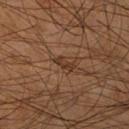| feature | finding |
|---|---|
| diameter | ≈2.5 mm |
| tile lighting | cross-polarized illumination |
| body site | the right lower leg |
| image-analysis metrics | an average lesion color of about L≈31 a*≈17 b*≈27 (CIELAB), about 8 CIELAB-L* units darker than the surrounding skin, and a normalized lesion–skin contrast near 8; a border-irregularity rating of about 4.5/10 and a peripheral color-asymmetry measure near 0; a nevus-likeness score of about 0/100 |
| patient | male, aged around 55 |
| image | ~15 mm tile from a whole-body skin photo |